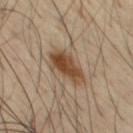Acquisition and patient details:
On the chest. An algorithmic analysis of the crop reported a mean CIELAB color near L≈44 a*≈16 b*≈30, about 12 CIELAB-L* units darker than the surrounding skin, and a normalized border contrast of about 10. The software also gave a border-irregularity rating of about 3/10. And it measured a nevus-likeness score of about 95/100 and lesion-presence confidence of about 100/100. Measured at roughly 4.5 mm in maximum diameter. A region of skin cropped from a whole-body photographic capture, roughly 15 mm wide. The subject is a male about 60 years old.